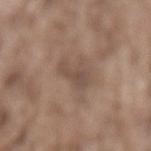Cropped from a whole-body photographic skin survey; the tile spans about 15 mm.
Imaged with white-light lighting.
Longest diameter approximately 4 mm.
The lesion is on the lower back.
A male subject aged around 75.
Automated image analysis of the tile measured a lesion area of about 4 mm², a shape eccentricity near 0.95, and a symmetry-axis asymmetry near 0.45. The analysis additionally found border irregularity of about 6 on a 0–10 scale, internal color variation of about 1 on a 0–10 scale, and radial color variation of about 0.